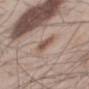{
  "biopsy_status": "not biopsied; imaged during a skin examination",
  "automated_metrics": {
    "cielab_L": 53,
    "cielab_a": 17,
    "cielab_b": 24,
    "vs_skin_darker_L": 11.0,
    "vs_skin_contrast_norm": 7.5,
    "border_irregularity_0_10": 4.0,
    "color_variation_0_10": 2.5,
    "peripheral_color_asymmetry": 0.5
  },
  "lesion_size": {
    "long_diameter_mm_approx": 3.5
  },
  "site": "mid back",
  "image": {
    "source": "total-body photography crop",
    "field_of_view_mm": 15
  },
  "patient": {
    "sex": "male",
    "age_approx": 55
  },
  "lighting": "white-light"
}